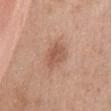<lesion>
<biopsy_status>not biopsied; imaged during a skin examination</biopsy_status>
<image>
  <source>total-body photography crop</source>
  <field_of_view_mm>15</field_of_view_mm>
</image>
<patient>
  <sex>female</sex>
  <age_approx>50</age_approx>
</patient>
<site>abdomen</site>
<automated_metrics>
  <nevus_likeness_0_100>60</nevus_likeness_0_100>
  <lesion_detection_confidence_0_100>100</lesion_detection_confidence_0_100>
</automated_metrics>
<lighting>white-light</lighting>
</lesion>